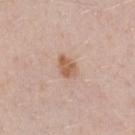The lesion was tiled from a total-body skin photograph and was not biopsied.
Captured under white-light illumination.
Cropped from a total-body skin-imaging series; the visible field is about 15 mm.
A male patient, roughly 40 years of age.
Located on the chest.